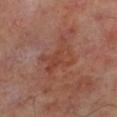No biopsy was performed on this lesion — it was imaged during a full skin examination and was not determined to be concerning. A male patient, about 50 years old. A roughly 15 mm field-of-view crop from a total-body skin photograph. On the left lower leg.